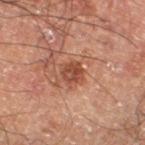The lesion was tiled from a total-body skin photograph and was not biopsied.
Cropped from a total-body skin-imaging series; the visible field is about 15 mm.
The lesion-visualizer software estimated a lesion area of about 5 mm² and two-axis asymmetry of about 0.15. It also reported an average lesion color of about L≈43 a*≈23 b*≈29 (CIELAB) and roughly 10 lightness units darker than nearby skin. It also reported a border-irregularity rating of about 1.5/10, internal color variation of about 4.5 on a 0–10 scale, and a peripheral color-asymmetry measure near 1.5. The analysis additionally found a classifier nevus-likeness of about 70/100 and a lesion-detection confidence of about 100/100.
Imaged with cross-polarized lighting.
The patient is a male about 60 years old.
Located on the right thigh.